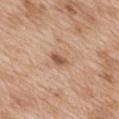follow-up: no biopsy performed (imaged during a skin exam)
TBP lesion metrics: a lesion area of about 2.5 mm² and an eccentricity of roughly 0.85; lesion-presence confidence of about 100/100
site: the mid back
size: ≈2.5 mm
subject: female, about 40 years old
illumination: white-light illumination
imaging modality: ~15 mm tile from a whole-body skin photo